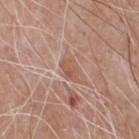The lesion was photographed on a routine skin check and not biopsied; there is no pathology result.
Automated tile analysis of the lesion measured an average lesion color of about L≈55 a*≈21 b*≈29 (CIELAB), roughly 7 lightness units darker than nearby skin, and a normalized border contrast of about 6. It also reported a nevus-likeness score of about 0/100 and a detector confidence of about 60 out of 100 that the crop contains a lesion.
The recorded lesion diameter is about 2.5 mm.
This is a white-light tile.
A region of skin cropped from a whole-body photographic capture, roughly 15 mm wide.
Located on the chest.
A male patient aged approximately 65.